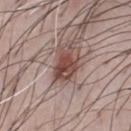This lesion was catalogued during total-body skin photography and was not selected for biopsy. Cropped from a total-body skin-imaging series; the visible field is about 15 mm. This is a white-light tile. The lesion-visualizer software estimated a footprint of about 8.5 mm², an outline eccentricity of about 0.8 (0 = round, 1 = elongated), and a symmetry-axis asymmetry near 0.35. The analysis additionally found a lesion color around L≈46 a*≈20 b*≈23 in CIELAB, roughly 13 lightness units darker than nearby skin, and a lesion-to-skin contrast of about 10 (normalized; higher = more distinct). The software also gave a border-irregularity index near 3.5/10, internal color variation of about 6 on a 0–10 scale, and radial color variation of about 2.5. And it measured a nevus-likeness score of about 85/100. The subject is a male in their 50s. From the chest. The recorded lesion diameter is about 4.5 mm.